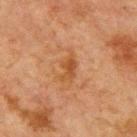Clinical impression:
Recorded during total-body skin imaging; not selected for excision or biopsy.
Image and clinical context:
About 4 mm across. This image is a 15 mm lesion crop taken from a total-body photograph. On the chest. Captured under cross-polarized illumination. An algorithmic analysis of the crop reported an area of roughly 6 mm², an eccentricity of roughly 0.9, and a shape-asymmetry score of about 0.4 (0 = symmetric). The analysis additionally found a lesion color around L≈42 a*≈20 b*≈33 in CIELAB, roughly 7 lightness units darker than nearby skin, and a normalized border contrast of about 6.5. The analysis additionally found a border-irregularity index near 5/10 and a color-variation rating of about 3/10. The patient is a male aged around 65.